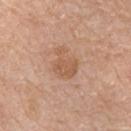<tbp_lesion>
  <biopsy_status>not biopsied; imaged during a skin examination</biopsy_status>
  <site>chest</site>
  <lighting>white-light</lighting>
  <image>
    <source>total-body photography crop</source>
    <field_of_view_mm>15</field_of_view_mm>
  </image>
  <patient>
    <sex>male</sex>
    <age_approx>60</age_approx>
  </patient>
</tbp_lesion>